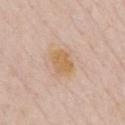This lesion was catalogued during total-body skin photography and was not selected for biopsy. The lesion is located on the front of the torso. This image is a 15 mm lesion crop taken from a total-body photograph. This is a white-light tile. A male subject about 75 years old. The total-body-photography lesion software estimated a footprint of about 7.5 mm², an outline eccentricity of about 0.6 (0 = round, 1 = elongated), and two-axis asymmetry of about 0.2. The analysis additionally found an average lesion color of about L≈64 a*≈16 b*≈37 (CIELAB), about 7 CIELAB-L* units darker than the surrounding skin, and a normalized border contrast of about 8.5.